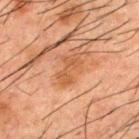Clinical impression: The lesion was tiled from a total-body skin photograph and was not biopsied.